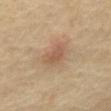follow-up: no biopsy performed (imaged during a skin exam); subject: male, in their 70s; anatomic site: the mid back; lesion size: about 3.5 mm; acquisition: total-body-photography crop, ~15 mm field of view.